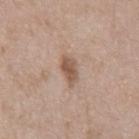Findings:
- biopsy status: total-body-photography surveillance lesion; no biopsy
- location: the chest
- diameter: about 3.5 mm
- tile lighting: white-light
- TBP lesion metrics: a lesion color around L≈54 a*≈18 b*≈28 in CIELAB, about 11 CIELAB-L* units darker than the surrounding skin, and a normalized border contrast of about 8; border irregularity of about 3 on a 0–10 scale, a within-lesion color-variation index near 2/10, and radial color variation of about 0.5; a nevus-likeness score of about 50/100
- subject: male, aged 48 to 52
- image source: 15 mm crop, total-body photography